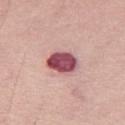Q: Was this lesion biopsied?
A: imaged on a skin check; not biopsied
Q: What is the lesion's diameter?
A: ~4 mm (longest diameter)
Q: Lesion location?
A: the right thigh
Q: How was this image acquired?
A: ~15 mm crop, total-body skin-cancer survey
Q: Who is the patient?
A: female, aged around 65
Q: Illumination type?
A: white-light
Q: Automated lesion metrics?
A: an automated nevus-likeness rating near 15 out of 100